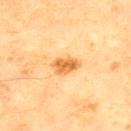Assessment: No biopsy was performed on this lesion — it was imaged during a full skin examination and was not determined to be concerning. Clinical summary: Imaged with cross-polarized lighting. Measured at roughly 3.5 mm in maximum diameter. A male subject, aged 63–67. Located on the chest. A close-up tile cropped from a whole-body skin photograph, about 15 mm across.